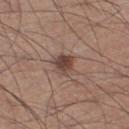{
  "biopsy_status": "not biopsied; imaged during a skin examination",
  "lesion_size": {
    "long_diameter_mm_approx": 3.0
  },
  "site": "right thigh",
  "lighting": "white-light",
  "patient": {
    "sex": "male",
    "age_approx": 55
  },
  "image": {
    "source": "total-body photography crop",
    "field_of_view_mm": 15
  }
}